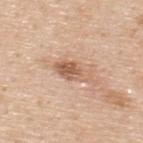Clinical impression:
Imaged during a routine full-body skin examination; the lesion was not biopsied and no histopathology is available.
Clinical summary:
Automated tile analysis of the lesion measured a footprint of about 6.5 mm², a shape eccentricity near 0.85, and two-axis asymmetry of about 0.2. And it measured an average lesion color of about L≈60 a*≈21 b*≈31 (CIELAB), roughly 12 lightness units darker than nearby skin, and a normalized border contrast of about 7.5. It also reported a border-irregularity rating of about 3/10, a within-lesion color-variation index near 4/10, and a peripheral color-asymmetry measure near 1.5. This is a white-light tile. The lesion is located on the upper back. Approximately 4 mm at its widest. Cropped from a total-body skin-imaging series; the visible field is about 15 mm. A male subject, roughly 50 years of age.